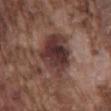Q: What is the lesion's diameter?
A: about 7.5 mm
Q: Patient demographics?
A: male, in their mid-70s
Q: How was this image acquired?
A: total-body-photography crop, ~15 mm field of view
Q: Where on the body is the lesion?
A: the mid back
Q: Illumination type?
A: white-light